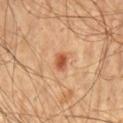The lesion was tiled from a total-body skin photograph and was not biopsied.
The lesion is located on the chest.
A male subject aged 53–57.
The lesion-visualizer software estimated about 12 CIELAB-L* units darker than the surrounding skin and a lesion-to-skin contrast of about 8 (normalized; higher = more distinct). It also reported a color-variation rating of about 3/10 and radial color variation of about 1. The software also gave a classifier nevus-likeness of about 95/100 and a lesion-detection confidence of about 100/100.
A 15 mm close-up extracted from a 3D total-body photography capture.
Approximately 2.5 mm at its widest.
The tile uses cross-polarized illumination.